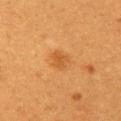Q: Is there a histopathology result?
A: imaged on a skin check; not biopsied
Q: Automated lesion metrics?
A: a shape eccentricity near 0.7 and a symmetry-axis asymmetry near 0.3; a nevus-likeness score of about 55/100 and a detector confidence of about 100 out of 100 that the crop contains a lesion
Q: How was this image acquired?
A: total-body-photography crop, ~15 mm field of view
Q: What are the patient's age and sex?
A: female, in their 30s
Q: Where on the body is the lesion?
A: the left upper arm